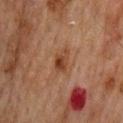Clinical impression: Captured during whole-body skin photography for melanoma surveillance; the lesion was not biopsied. Context: A male patient, aged approximately 70. Cropped from a whole-body photographic skin survey; the tile spans about 15 mm. On the back. This is a cross-polarized tile. The recorded lesion diameter is about 3 mm.